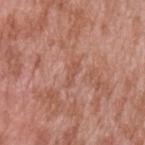| feature | finding |
|---|---|
| notes | catalogued during a skin exam; not biopsied |
| patient | male, in their 40s |
| tile lighting | white-light |
| lesion size | ≈3 mm |
| image | 15 mm crop, total-body photography |
| location | the left upper arm |